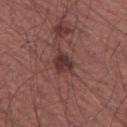This lesion was catalogued during total-body skin photography and was not selected for biopsy. The lesion's longest dimension is about 2.5 mm. An algorithmic analysis of the crop reported a lesion area of about 5 mm². A male subject roughly 45 years of age. A 15 mm close-up tile from a total-body photography series done for melanoma screening. Located on the right thigh.